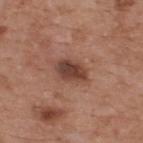Case summary:
• subject — male, aged around 55
• lesion diameter — ≈4 mm
• lighting — white-light illumination
• anatomic site — the upper back
• TBP lesion metrics — an eccentricity of roughly 0.75 and a shape-asymmetry score of about 0.25 (0 = symmetric); a border-irregularity index near 2/10, a within-lesion color-variation index near 5.5/10, and a peripheral color-asymmetry measure near 2
• image source — total-body-photography crop, ~15 mm field of view